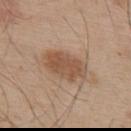Recorded during total-body skin imaging; not selected for excision or biopsy.
This image is a 15 mm lesion crop taken from a total-body photograph.
The tile uses white-light illumination.
The patient is a male aged 53–57.
The lesion-visualizer software estimated a footprint of about 12 mm², an eccentricity of roughly 0.8, and a shape-asymmetry score of about 0.15 (0 = symmetric). And it measured border irregularity of about 2 on a 0–10 scale, internal color variation of about 3 on a 0–10 scale, and peripheral color asymmetry of about 1. And it measured a nevus-likeness score of about 60/100 and a detector confidence of about 100 out of 100 that the crop contains a lesion.
The lesion is on the upper back.